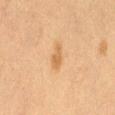Part of a total-body skin-imaging series; this lesion was reviewed on a skin check and was not flagged for biopsy. Located on the left thigh. A lesion tile, about 15 mm wide, cut from a 3D total-body photograph. A female patient, aged approximately 40. The recorded lesion diameter is about 3 mm. The lesion-visualizer software estimated border irregularity of about 2.5 on a 0–10 scale, a color-variation rating of about 1.5/10, and peripheral color asymmetry of about 0.5.